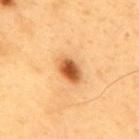| key | value |
|---|---|
| follow-up | total-body-photography surveillance lesion; no biopsy |
| illumination | cross-polarized |
| patient | male, approximately 60 years of age |
| site | the back |
| lesion diameter | ≈4 mm |
| image source | ~15 mm tile from a whole-body skin photo |